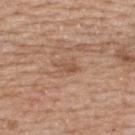biopsy status=total-body-photography surveillance lesion; no biopsy | image=~15 mm tile from a whole-body skin photo | body site=the upper back | subject=female, roughly 60 years of age | lesion diameter=~2.5 mm (longest diameter) | TBP lesion metrics=a border-irregularity index near 3/10; an automated nevus-likeness rating near 0 out of 100 and a detector confidence of about 100 out of 100 that the crop contains a lesion | illumination=white-light.